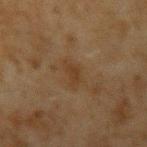Recorded during total-body skin imaging; not selected for excision or biopsy. From the left upper arm. A lesion tile, about 15 mm wide, cut from a 3D total-body photograph. About 3 mm across. Captured under cross-polarized illumination. A male patient approximately 45 years of age.